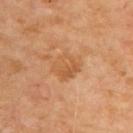Background:
The patient is a male approximately 70 years of age. Imaged with cross-polarized lighting. This image is a 15 mm lesion crop taken from a total-body photograph. The lesion is on the upper back. Measured at roughly 3.5 mm in maximum diameter. The lesion-visualizer software estimated a lesion area of about 9 mm² and an outline eccentricity of about 0.45 (0 = round, 1 = elongated). It also reported about 7 CIELAB-L* units darker than the surrounding skin and a normalized border contrast of about 5.5. It also reported a border-irregularity rating of about 2/10, a within-lesion color-variation index near 3.5/10, and a peripheral color-asymmetry measure near 1.5. The software also gave a nevus-likeness score of about 0/100.